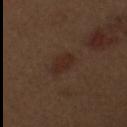Notes:
* notes — no biopsy performed (imaged during a skin exam)
* tile lighting — white-light illumination
* body site — the arm
* lesion size — about 2.5 mm
* imaging modality — ~15 mm tile from a whole-body skin photo
* patient — male, approximately 70 years of age